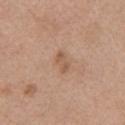Recorded during total-body skin imaging; not selected for excision or biopsy. A male subject, aged 58–62. The lesion is on the left upper arm. A 15 mm close-up extracted from a 3D total-body photography capture. Imaged with white-light lighting. The recorded lesion diameter is about 2.5 mm. The lesion-visualizer software estimated an average lesion color of about L≈56 a*≈19 b*≈31 (CIELAB), about 8 CIELAB-L* units darker than the surrounding skin, and a normalized lesion–skin contrast near 6.